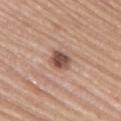Q: Was this lesion biopsied?
A: imaged on a skin check; not biopsied
Q: Who is the patient?
A: female, in their mid- to late 60s
Q: What kind of image is this?
A: total-body-photography crop, ~15 mm field of view
Q: What lighting was used for the tile?
A: white-light illumination
Q: What is the lesion's diameter?
A: ≈3 mm
Q: Where on the body is the lesion?
A: the upper back